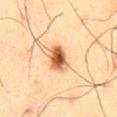Recorded during total-body skin imaging; not selected for excision or biopsy. The lesion is on the abdomen. Cropped from a total-body skin-imaging series; the visible field is about 15 mm. A male subject approximately 60 years of age. Measured at roughly 3.5 mm in maximum diameter. This is a cross-polarized tile.